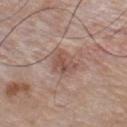Q: Was this lesion biopsied?
A: catalogued during a skin exam; not biopsied
Q: What are the patient's age and sex?
A: male, approximately 55 years of age
Q: What kind of image is this?
A: ~15 mm crop, total-body skin-cancer survey
Q: Lesion location?
A: the chest